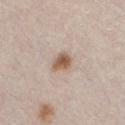Assessment: Recorded during total-body skin imaging; not selected for excision or biopsy. Acquisition and patient details: The lesion's longest dimension is about 2.5 mm. This image is a 15 mm lesion crop taken from a total-body photograph. A male patient aged 28 to 32. The lesion-visualizer software estimated a border-irregularity index near 2/10 and a color-variation rating of about 3.5/10. The software also gave a detector confidence of about 100 out of 100 that the crop contains a lesion. Captured under white-light illumination.